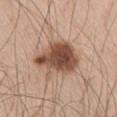Impression:
Part of a total-body skin-imaging series; this lesion was reviewed on a skin check and was not flagged for biopsy.
Background:
The total-body-photography lesion software estimated a border-irregularity rating of about 3/10, internal color variation of about 6.5 on a 0–10 scale, and peripheral color asymmetry of about 2. The analysis additionally found an automated nevus-likeness rating near 85 out of 100 and a detector confidence of about 100 out of 100 that the crop contains a lesion. From the right thigh. A roughly 15 mm field-of-view crop from a total-body skin photograph. About 6.5 mm across. Imaged with white-light lighting. A male subject aged 58–62.